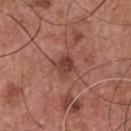{"biopsy_status": "not biopsied; imaged during a skin examination", "patient": {"sex": "male", "age_approx": 55}, "image": {"source": "total-body photography crop", "field_of_view_mm": 15}, "lighting": "white-light", "lesion_size": {"long_diameter_mm_approx": 2.5}, "site": "chest", "automated_metrics": {"cielab_L": 42, "cielab_a": 24, "cielab_b": 26, "vs_skin_darker_L": 10.0, "vs_skin_contrast_norm": 7.5, "color_variation_0_10": 4.0, "lesion_detection_confidence_0_100": 100}}